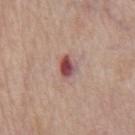Captured during whole-body skin photography for melanoma surveillance; the lesion was not biopsied. A male subject aged approximately 65. A region of skin cropped from a whole-body photographic capture, roughly 15 mm wide. Automated image analysis of the tile measured a lesion area of about 4.5 mm², an outline eccentricity of about 0.6 (0 = round, 1 = elongated), and two-axis asymmetry of about 0.3. It also reported a border-irregularity rating of about 2.5/10, a color-variation rating of about 7.5/10, and radial color variation of about 2. Located on the chest.